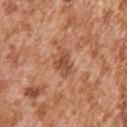Impression: Recorded during total-body skin imaging; not selected for excision or biopsy. Background: A male subject, aged 43 to 47. Captured under white-light illumination. About 3 mm across. A roughly 15 mm field-of-view crop from a total-body skin photograph. The lesion is located on the right upper arm.